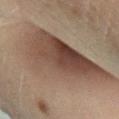Impression:
The lesion was photographed on a routine skin check and not biopsied; there is no pathology result.
Image and clinical context:
The tile uses cross-polarized illumination. The subject is a male aged 68 to 72. A region of skin cropped from a whole-body photographic capture, roughly 15 mm wide. Located on the leg. The lesion's longest dimension is about 14 mm. An algorithmic analysis of the crop reported a lesion area of about 55 mm², an outline eccentricity of about 0.9 (0 = round, 1 = elongated), and a symmetry-axis asymmetry near 0.35. The analysis additionally found a border-irregularity index near 5/10 and internal color variation of about 7 on a 0–10 scale.